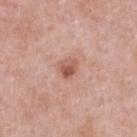Q: Is there a histopathology result?
A: no biopsy performed (imaged during a skin exam)
Q: How was this image acquired?
A: total-body-photography crop, ~15 mm field of view
Q: Where on the body is the lesion?
A: the upper back
Q: What are the patient's age and sex?
A: male, aged 53–57